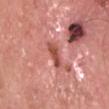<case>
  <biopsy_status>not biopsied; imaged during a skin examination</biopsy_status>
  <image>
    <source>total-body photography crop</source>
    <field_of_view_mm>15</field_of_view_mm>
  </image>
  <site>head or neck</site>
  <patient>
    <sex>male</sex>
    <age_approx>60</age_approx>
  </patient>
</case>